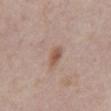The lesion was photographed on a routine skin check and not biopsied; there is no pathology result. A male subject aged around 55. Automated image analysis of the tile measured a footprint of about 3.5 mm² and an eccentricity of roughly 0.8. The analysis additionally found a mean CIELAB color near L≈54 a*≈19 b*≈27 and a normalized lesion–skin contrast near 7.5. And it measured border irregularity of about 2 on a 0–10 scale and a peripheral color-asymmetry measure near 1. The lesion's longest dimension is about 2.5 mm. A 15 mm crop from a total-body photograph taken for skin-cancer surveillance. The lesion is located on the abdomen.